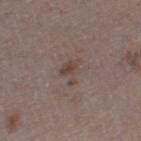biopsy status: no biopsy performed (imaged during a skin exam); patient: male, roughly 75 years of age; anatomic site: the right thigh; acquisition: total-body-photography crop, ~15 mm field of view; diameter: about 3.5 mm.